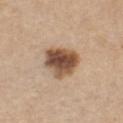{"lesion_size": {"long_diameter_mm_approx": 4.5}, "patient": {"sex": "female", "age_approx": 45}, "lighting": "white-light", "site": "front of the torso", "image": {"source": "total-body photography crop", "field_of_view_mm": 15}}